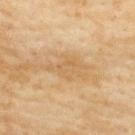Part of a total-body skin-imaging series; this lesion was reviewed on a skin check and was not flagged for biopsy. From the back. A female subject aged 58 to 62. A lesion tile, about 15 mm wide, cut from a 3D total-body photograph. Approximately 4.5 mm at its widest. This is a cross-polarized tile. An algorithmic analysis of the crop reported a footprint of about 7 mm², an eccentricity of roughly 0.85, and two-axis asymmetry of about 0.45. The analysis additionally found an average lesion color of about L≈58 a*≈16 b*≈37 (CIELAB), a lesion–skin lightness drop of about 6, and a lesion-to-skin contrast of about 5 (normalized; higher = more distinct). The software also gave a detector confidence of about 65 out of 100 that the crop contains a lesion.